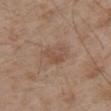Q: Was a biopsy performed?
A: catalogued during a skin exam; not biopsied
Q: What kind of image is this?
A: ~15 mm crop, total-body skin-cancer survey
Q: What is the anatomic site?
A: the right upper arm
Q: Who is the patient?
A: male, aged 53 to 57